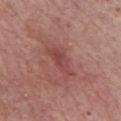  biopsy_status: not biopsied; imaged during a skin examination
  lesion_size:
    long_diameter_mm_approx: 5.0
  patient:
    sex: male
    age_approx: 75
  image:
    source: total-body photography crop
    field_of_view_mm: 15
  site: chest